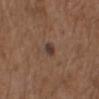Recorded during total-body skin imaging; not selected for excision or biopsy. Imaged with white-light lighting. The patient is a male approximately 75 years of age. The lesion is located on the upper back. Measured at roughly 2 mm in maximum diameter. Cropped from a total-body skin-imaging series; the visible field is about 15 mm.